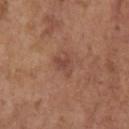biopsy status: total-body-photography surveillance lesion; no biopsy | lesion size: ≈3 mm | body site: the left upper arm | subject: female, in their mid-60s | image: ~15 mm tile from a whole-body skin photo | lighting: white-light.